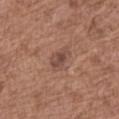workup: no biopsy performed (imaged during a skin exam) | diameter: ≈3 mm | patient: female, in their mid-70s | image: 15 mm crop, total-body photography | body site: the leg.